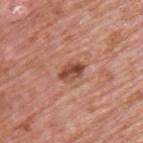Findings:
• workup · imaged on a skin check; not biopsied
• image · ~15 mm crop, total-body skin-cancer survey
• illumination · white-light
• lesion diameter · ~3 mm (longest diameter)
• subject · male, aged 73 to 77
• location · the upper back
• image-analysis metrics · a within-lesion color-variation index near 5.5/10 and peripheral color asymmetry of about 2; a nevus-likeness score of about 70/100 and a lesion-detection confidence of about 100/100